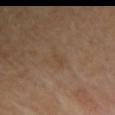This lesion was catalogued during total-body skin photography and was not selected for biopsy. The patient is a female aged 68 to 72. From the left upper arm. A 15 mm crop from a total-body photograph taken for skin-cancer surveillance.